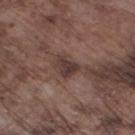biopsy_status: not biopsied; imaged during a skin examination
patient:
  sex: male
  age_approx: 75
lesion_size:
  long_diameter_mm_approx: 2.5
image:
  source: total-body photography crop
  field_of_view_mm: 15
site: left lower leg